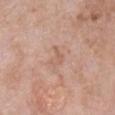workup: catalogued during a skin exam; not biopsied
lighting: white-light illumination
imaging modality: ~15 mm tile from a whole-body skin photo
subject: female, aged approximately 75
site: the chest
lesion size: ≈2.5 mm